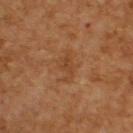Clinical impression: Recorded during total-body skin imaging; not selected for excision or biopsy. Image and clinical context: A roughly 15 mm field-of-view crop from a total-body skin photograph. A male patient, about 60 years old. Located on the upper back. The tile uses cross-polarized illumination.